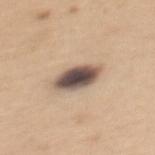Captured during whole-body skin photography for melanoma surveillance; the lesion was not biopsied.
Automated image analysis of the tile measured a symmetry-axis asymmetry near 0.15. It also reported a classifier nevus-likeness of about 35/100 and a lesion-detection confidence of about 100/100.
A female subject aged 28–32.
This is a white-light tile.
The recorded lesion diameter is about 4.5 mm.
Cropped from a whole-body photographic skin survey; the tile spans about 15 mm.
The lesion is on the mid back.